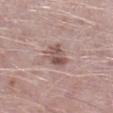Assessment:
Part of a total-body skin-imaging series; this lesion was reviewed on a skin check and was not flagged for biopsy.
Acquisition and patient details:
A lesion tile, about 15 mm wide, cut from a 3D total-body photograph. A male subject aged 48 to 52. From the leg.